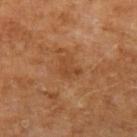biopsy status — no biopsy performed (imaged during a skin exam); diameter — ≈3 mm; subject — male, approximately 55 years of age; image source — total-body-photography crop, ~15 mm field of view; lighting — cross-polarized illumination; anatomic site — the arm.